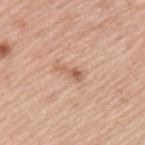Case summary:
• workup — no biopsy performed (imaged during a skin exam)
• patient — male, about 45 years old
• lighting — white-light illumination
• image — ~15 mm crop, total-body skin-cancer survey
• site — the back
• lesion diameter — ≈3 mm
• image-analysis metrics — an eccentricity of roughly 0.95 and a shape-asymmetry score of about 0.55 (0 = symmetric); a mean CIELAB color near L≈60 a*≈22 b*≈32; a border-irregularity rating of about 6.5/10 and a within-lesion color-variation index near 0/10